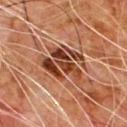Notes:
- notes · no biopsy performed (imaged during a skin exam)
- location · the chest
- image · total-body-photography crop, ~15 mm field of view
- lesion size · ≈5.5 mm
- patient · male, about 80 years old
- tile lighting · cross-polarized